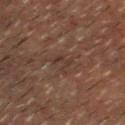Q: Is there a histopathology result?
A: imaged on a skin check; not biopsied
Q: What is the anatomic site?
A: the head or neck
Q: How large is the lesion?
A: about 2.5 mm
Q: Patient demographics?
A: male, roughly 60 years of age
Q: What is the imaging modality?
A: ~15 mm tile from a whole-body skin photo
Q: How was the tile lit?
A: cross-polarized